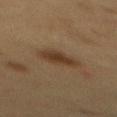follow-up: imaged on a skin check; not biopsied | diameter: ≈4.5 mm | image source: ~15 mm tile from a whole-body skin photo | location: the mid back | patient: female, aged around 50.